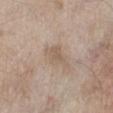Impression: The lesion was tiled from a total-body skin photograph and was not biopsied. Clinical summary: An algorithmic analysis of the crop reported a border-irregularity rating of about 3.5/10, internal color variation of about 2 on a 0–10 scale, and peripheral color asymmetry of about 0.5. And it measured a classifier nevus-likeness of about 5/100. The tile uses white-light illumination. Located on the left lower leg. Measured at roughly 4 mm in maximum diameter. A 15 mm close-up extracted from a 3D total-body photography capture. The subject is a male aged approximately 60.